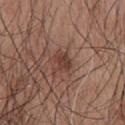Clinical impression: The lesion was photographed on a routine skin check and not biopsied; there is no pathology result. Background: A 15 mm crop from a total-body photograph taken for skin-cancer surveillance. From the upper back. About 2.5 mm across. This is a white-light tile. A male subject, aged around 50.